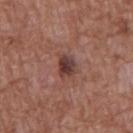- image: 15 mm crop, total-body photography
- subject: male, aged approximately 75
- lighting: white-light
- anatomic site: the chest